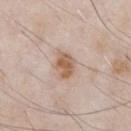| key | value |
|---|---|
| workup | total-body-photography surveillance lesion; no biopsy |
| body site | the chest |
| imaging modality | ~15 mm crop, total-body skin-cancer survey |
| subject | male, about 55 years old |
| lesion size | about 3 mm |